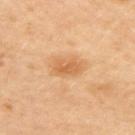workup = catalogued during a skin exam; not biopsied
subject = female, aged 38 to 42
automated lesion analysis = a border-irregularity index near 2/10, internal color variation of about 3 on a 0–10 scale, and a peripheral color-asymmetry measure near 1
body site = the left arm
imaging modality = total-body-photography crop, ~15 mm field of view